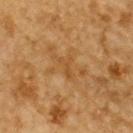- follow-up — total-body-photography surveillance lesion; no biopsy
- subject — male, aged 83 or older
- illumination — cross-polarized illumination
- body site — the upper back
- acquisition — ~15 mm crop, total-body skin-cancer survey
- automated lesion analysis — an eccentricity of roughly 0.85 and a symmetry-axis asymmetry near 0.5; a border-irregularity rating of about 5.5/10, internal color variation of about 0 on a 0–10 scale, and a peripheral color-asymmetry measure near 0; a classifier nevus-likeness of about 0/100 and lesion-presence confidence of about 100/100